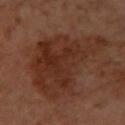| feature | finding |
|---|---|
| follow-up | total-body-photography surveillance lesion; no biopsy |
| location | the left forearm |
| lighting | cross-polarized |
| imaging modality | total-body-photography crop, ~15 mm field of view |
| subject | female, roughly 50 years of age |